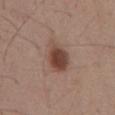notes: catalogued during a skin exam; not biopsied
automated lesion analysis: a lesion area of about 10 mm² and two-axis asymmetry of about 0.2; a mean CIELAB color near L≈44 a*≈19 b*≈25, a lesion–skin lightness drop of about 12, and a lesion-to-skin contrast of about 9.5 (normalized; higher = more distinct)
image source: 15 mm crop, total-body photography
patient: male, about 55 years old
location: the front of the torso